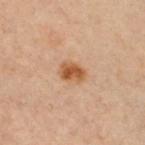The lesion was tiled from a total-body skin photograph and was not biopsied. The patient is a female approximately 30 years of age. On the chest. A 15 mm close-up tile from a total-body photography series done for melanoma screening.